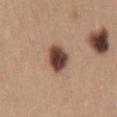Clinical impression:
Recorded during total-body skin imaging; not selected for excision or biopsy.
Background:
Imaged with white-light lighting. The lesion is on the abdomen. Cropped from a total-body skin-imaging series; the visible field is about 15 mm. Approximately 3.5 mm at its widest. The patient is a female in their mid- to late 40s.